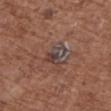The lesion was tiled from a total-body skin photograph and was not biopsied. A female subject, aged 73–77. Longest diameter approximately 2.5 mm. A lesion tile, about 15 mm wide, cut from a 3D total-body photograph. From the upper back. Captured under white-light illumination. Automated image analysis of the tile measured a footprint of about 5.5 mm², an eccentricity of roughly 0.55, and a shape-asymmetry score of about 0.4 (0 = symmetric). And it measured a border-irregularity rating of about 4/10, internal color variation of about 9.5 on a 0–10 scale, and radial color variation of about 3. It also reported a classifier nevus-likeness of about 0/100.